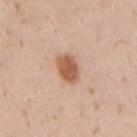{"biopsy_status": "not biopsied; imaged during a skin examination", "site": "right upper arm", "patient": {"sex": "male", "age_approx": 35}, "image": {"source": "total-body photography crop", "field_of_view_mm": 15}, "lesion_size": {"long_diameter_mm_approx": 3.5}}